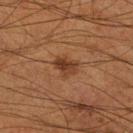Impression: Recorded during total-body skin imaging; not selected for excision or biopsy. Context: A male patient, roughly 55 years of age. The lesion is on the right lower leg. A close-up tile cropped from a whole-body skin photograph, about 15 mm across. The tile uses cross-polarized illumination.